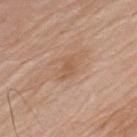notes: total-body-photography surveillance lesion; no biopsy